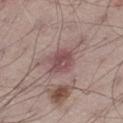Case summary:
* location: the left thigh
* patient: male, about 70 years old
* tile lighting: white-light
* lesion size: ≈3.5 mm
* TBP lesion metrics: an area of roughly 8 mm² and an eccentricity of roughly 0.25; an average lesion color of about L≈49 a*≈21 b*≈18 (CIELAB) and a lesion–skin lightness drop of about 10; a border-irregularity rating of about 2/10, a within-lesion color-variation index near 3.5/10, and peripheral color asymmetry of about 1.5
* image source: 15 mm crop, total-body photography